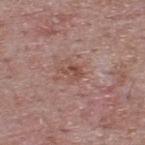Q: Illumination type?
A: white-light
Q: Patient demographics?
A: male, aged around 65
Q: Lesion location?
A: the upper back
Q: What is the imaging modality?
A: total-body-photography crop, ~15 mm field of view
Q: Automated lesion metrics?
A: roughly 8 lightness units darker than nearby skin and a lesion-to-skin contrast of about 6.5 (normalized; higher = more distinct); a border-irregularity rating of about 4/10, a color-variation rating of about 3/10, and peripheral color asymmetry of about 1
Q: What is the lesion's diameter?
A: ≈2.5 mm